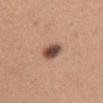{"biopsy_status": "not biopsied; imaged during a skin examination", "lighting": "white-light", "lesion_size": {"long_diameter_mm_approx": 2.5}, "patient": {"sex": "female", "age_approx": 30}, "site": "abdomen", "image": {"source": "total-body photography crop", "field_of_view_mm": 15}}